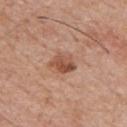– notes · imaged on a skin check; not biopsied